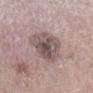follow-up: catalogued during a skin exam; not biopsied | location: the leg | size: about 5 mm | automated metrics: a footprint of about 17 mm², an outline eccentricity of about 0.35 (0 = round, 1 = elongated), and two-axis asymmetry of about 0.25; an average lesion color of about L≈52 a*≈15 b*≈17 (CIELAB) and a lesion-to-skin contrast of about 8.5 (normalized; higher = more distinct) | imaging modality: 15 mm crop, total-body photography | subject: male, roughly 65 years of age.